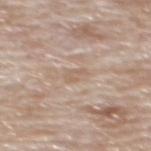notes — imaged on a skin check; not biopsied
location — the mid back
tile lighting — white-light illumination
imaging modality — total-body-photography crop, ~15 mm field of view
automated lesion analysis — a footprint of about 2.5 mm², an outline eccentricity of about 0.9 (0 = round, 1 = elongated), and a shape-asymmetry score of about 0.3 (0 = symmetric); a lesion color around L≈60 a*≈15 b*≈28 in CIELAB, roughly 7 lightness units darker than nearby skin, and a normalized lesion–skin contrast near 5; a color-variation rating of about 0/10
size — ~2.5 mm (longest diameter)
subject — male, aged around 80